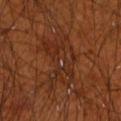Q: What are the patient's age and sex?
A: male, aged 68 to 72
Q: What did automated image analysis measure?
A: a mean CIELAB color near L≈28 a*≈22 b*≈29, roughly 5 lightness units darker than nearby skin, and a lesion-to-skin contrast of about 5.5 (normalized; higher = more distinct)
Q: Lesion location?
A: the arm
Q: Lesion size?
A: about 6.5 mm
Q: How was this image acquired?
A: ~15 mm tile from a whole-body skin photo